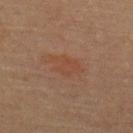Assessment:
Part of a total-body skin-imaging series; this lesion was reviewed on a skin check and was not flagged for biopsy.
Background:
A 15 mm crop from a total-body photograph taken for skin-cancer surveillance. A female patient, aged 63–67. On the upper back. The total-body-photography lesion software estimated border irregularity of about 3 on a 0–10 scale, internal color variation of about 1.5 on a 0–10 scale, and a peripheral color-asymmetry measure near 0.5. Approximately 4 mm at its widest.